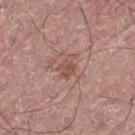{
  "biopsy_status": "not biopsied; imaged during a skin examination",
  "image": {
    "source": "total-body photography crop",
    "field_of_view_mm": 15
  },
  "site": "left thigh",
  "automated_metrics": {
    "area_mm2_approx": 4.0,
    "eccentricity": 0.75,
    "shape_asymmetry": 0.25,
    "cielab_L": 51,
    "cielab_a": 21,
    "cielab_b": 25,
    "vs_skin_darker_L": 8.0,
    "vs_skin_contrast_norm": 6.0
  },
  "patient": {
    "sex": "male",
    "age_approx": 75
  }
}